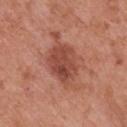<record>
<biopsy_status>not biopsied; imaged during a skin examination</biopsy_status>
<lighting>white-light</lighting>
<patient>
  <sex>male</sex>
  <age_approx>70</age_approx>
</patient>
<lesion_size>
  <long_diameter_mm_approx>5.0</long_diameter_mm_approx>
</lesion_size>
<image>
  <source>total-body photography crop</source>
  <field_of_view_mm>15</field_of_view_mm>
</image>
<automated_metrics>
  <area_mm2_approx>14.0</area_mm2_approx>
  <shape_asymmetry>0.15</shape_asymmetry>
  <color_variation_0_10>4.5</color_variation_0_10>
  <nevus_likeness_0_100>45</nevus_likeness_0_100>
  <lesion_detection_confidence_0_100>100</lesion_detection_confidence_0_100>
</automated_metrics>
<site>mid back</site>
</record>